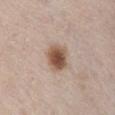Findings:
• follow-up · total-body-photography surveillance lesion; no biopsy
• lesion size · ≈3.5 mm
• anatomic site · the chest
• image · ~15 mm tile from a whole-body skin photo
• tile lighting · white-light
• patient · male, in their mid-20s